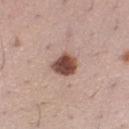The lesion was tiled from a total-body skin photograph and was not biopsied. Automated tile analysis of the lesion measured an area of roughly 7 mm² and a shape-asymmetry score of about 0.25 (0 = symmetric). A region of skin cropped from a whole-body photographic capture, roughly 15 mm wide. This is a white-light tile. From the right lower leg. The patient is a female approximately 35 years of age. The lesion's longest dimension is about 3 mm.